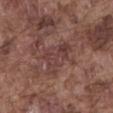Captured during whole-body skin photography for melanoma surveillance; the lesion was not biopsied. A male patient, approximately 75 years of age. Approximately 5 mm at its widest. A region of skin cropped from a whole-body photographic capture, roughly 15 mm wide. On the abdomen. The lesion-visualizer software estimated an outline eccentricity of about 0.8 (0 = round, 1 = elongated). The software also gave a lesion color around L≈40 a*≈21 b*≈21 in CIELAB, a lesion–skin lightness drop of about 6, and a normalized lesion–skin contrast near 6.